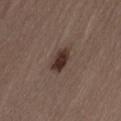Clinical impression:
Part of a total-body skin-imaging series; this lesion was reviewed on a skin check and was not flagged for biopsy.
Clinical summary:
A close-up tile cropped from a whole-body skin photograph, about 15 mm across. A female subject approximately 30 years of age. Longest diameter approximately 3.5 mm. From the lower back.